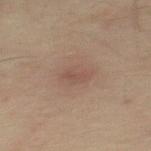Assessment: Captured during whole-body skin photography for melanoma surveillance; the lesion was not biopsied. Clinical summary: From the mid back. The subject is a male about 50 years old. A lesion tile, about 15 mm wide, cut from a 3D total-body photograph.